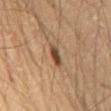Cropped from a whole-body photographic skin survey; the tile spans about 15 mm.
Measured at roughly 2.5 mm in maximum diameter.
Captured under cross-polarized illumination.
A male patient, roughly 55 years of age.
Located on the mid back.
An algorithmic analysis of the crop reported a lesion area of about 3.5 mm² and two-axis asymmetry of about 0.2. The analysis additionally found a lesion color around L≈44 a*≈19 b*≈30 in CIELAB, roughly 13 lightness units darker than nearby skin, and a normalized border contrast of about 10. The software also gave border irregularity of about 2 on a 0–10 scale and radial color variation of about 2.5. And it measured a classifier nevus-likeness of about 100/100 and a detector confidence of about 100 out of 100 that the crop contains a lesion.